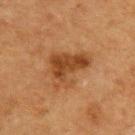Clinical impression: No biopsy was performed on this lesion — it was imaged during a full skin examination and was not determined to be concerning.